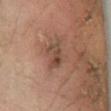notes = total-body-photography surveillance lesion; no biopsy
size = ≈3 mm
site = the right lower leg
subject = female, approximately 65 years of age
image source = ~15 mm tile from a whole-body skin photo